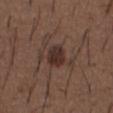Part of a total-body skin-imaging series; this lesion was reviewed on a skin check and was not flagged for biopsy. A roughly 15 mm field-of-view crop from a total-body skin photograph. The subject is a male aged 48–52. An algorithmic analysis of the crop reported a lesion area of about 5.5 mm², an eccentricity of roughly 0.5, and two-axis asymmetry of about 0.15. The analysis additionally found a lesion color around L≈30 a*≈17 b*≈20 in CIELAB, roughly 10 lightness units darker than nearby skin, and a normalized lesion–skin contrast near 9.5. The software also gave a within-lesion color-variation index near 2.5/10 and a peripheral color-asymmetry measure near 1. It also reported a classifier nevus-likeness of about 75/100 and a lesion-detection confidence of about 100/100. Approximately 2.5 mm at its widest. From the chest. Imaged with white-light lighting.